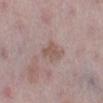Assessment: Captured during whole-body skin photography for melanoma surveillance; the lesion was not biopsied. Background: Imaged with white-light lighting. Longest diameter approximately 3 mm. The lesion is located on the left lower leg. A female subject approximately 50 years of age. A close-up tile cropped from a whole-body skin photograph, about 15 mm across.